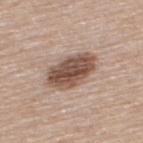The lesion was tiled from a total-body skin photograph and was not biopsied.